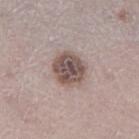Impression: The lesion was tiled from a total-body skin photograph and was not biopsied. Background: Located on the leg. The subject is a female aged 48–52. Captured under white-light illumination. Automated image analysis of the tile measured a lesion color around L≈51 a*≈15 b*≈20 in CIELAB, about 14 CIELAB-L* units darker than the surrounding skin, and a lesion-to-skin contrast of about 9.5 (normalized; higher = more distinct). And it measured an automated nevus-likeness rating near 10 out of 100. A 15 mm close-up tile from a total-body photography series done for melanoma screening. About 4 mm across.